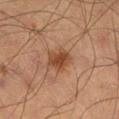follow-up = imaged on a skin check; not biopsied | subject = male, roughly 65 years of age | site = the left thigh | image source = ~15 mm tile from a whole-body skin photo.